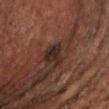Notes:
– biopsy status — catalogued during a skin exam; not biopsied
– illumination — cross-polarized illumination
– location — the abdomen
– automated lesion analysis — an area of roughly 5.5 mm², an eccentricity of roughly 0.65, and two-axis asymmetry of about 0.4; border irregularity of about 3.5 on a 0–10 scale and peripheral color asymmetry of about 1
– size — ≈3 mm
– patient — male, roughly 50 years of age
– imaging modality — 15 mm crop, total-body photography